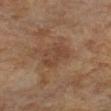Assessment:
Imaged during a routine full-body skin examination; the lesion was not biopsied and no histopathology is available.
Acquisition and patient details:
The recorded lesion diameter is about 3.5 mm. Cropped from a total-body skin-imaging series; the visible field is about 15 mm. The tile uses cross-polarized illumination. Located on the leg. A female subject approximately 70 years of age.